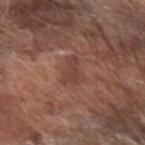{"biopsy_status": "not biopsied; imaged during a skin examination", "site": "arm", "automated_metrics": {"area_mm2_approx": 5.5, "eccentricity": 0.85, "shape_asymmetry": 0.45, "cielab_L": 42, "cielab_a": 21, "cielab_b": 25, "vs_skin_darker_L": 7.0, "vs_skin_contrast_norm": 5.5, "border_irregularity_0_10": 5.0, "color_variation_0_10": 1.5, "peripheral_color_asymmetry": 0.5, "nevus_likeness_0_100": 0}, "lighting": "white-light", "image": {"source": "total-body photography crop", "field_of_view_mm": 15}, "patient": {"sex": "male", "age_approx": 70}, "lesion_size": {"long_diameter_mm_approx": 4.0}}